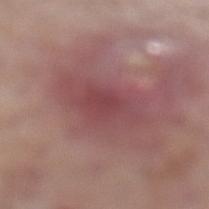Assessment:
Part of a total-body skin-imaging series; this lesion was reviewed on a skin check and was not flagged for biopsy.
Background:
From the left lower leg. A male patient about 80 years old. Automated image analysis of the tile measured a border-irregularity rating of about 3.5/10 and radial color variation of about 1. Cropped from a whole-body photographic skin survey; the tile spans about 15 mm. Approximately 7 mm at its widest.